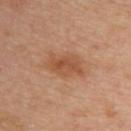Assessment:
Part of a total-body skin-imaging series; this lesion was reviewed on a skin check and was not flagged for biopsy.
Background:
A female patient aged 38–42. The lesion is on the upper back. Captured under cross-polarized illumination. A region of skin cropped from a whole-body photographic capture, roughly 15 mm wide. Measured at roughly 5 mm in maximum diameter.